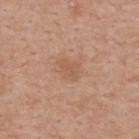  biopsy_status: not biopsied; imaged during a skin examination
  patient:
    sex: male
    age_approx: 60
  lighting: white-light
  lesion_size:
    long_diameter_mm_approx: 3.0
  image:
    source: total-body photography crop
    field_of_view_mm: 15
  site: upper back